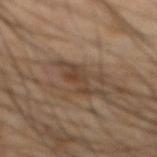Impression:
Imaged during a routine full-body skin examination; the lesion was not biopsied and no histopathology is available.
Image and clinical context:
The total-body-photography lesion software estimated an outline eccentricity of about 0.9 (0 = round, 1 = elongated). It also reported a lesion color around L≈38 a*≈14 b*≈26 in CIELAB, about 8 CIELAB-L* units darker than the surrounding skin, and a normalized lesion–skin contrast near 7. The subject is a male about 65 years old. A close-up tile cropped from a whole-body skin photograph, about 15 mm across. Longest diameter approximately 5 mm. The lesion is on the front of the torso.